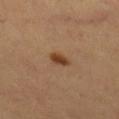A 15 mm crop from a total-body photograph taken for skin-cancer surveillance. A female patient approximately 60 years of age. The lesion is located on the right lower leg.